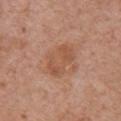follow-up=no biopsy performed (imaged during a skin exam) | imaging modality=~15 mm tile from a whole-body skin photo | anatomic site=the chest | size=≈4.5 mm | subject=male, aged 78 to 82 | image-analysis metrics=an area of roughly 10 mm², a shape eccentricity near 0.8, and two-axis asymmetry of about 0.2; a lesion–skin lightness drop of about 7 and a normalized border contrast of about 6; a color-variation rating of about 3.5/10 and radial color variation of about 1; a classifier nevus-likeness of about 5/100 and a lesion-detection confidence of about 100/100.